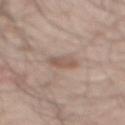<record>
  <biopsy_status>not biopsied; imaged during a skin examination</biopsy_status>
  <lighting>white-light</lighting>
  <lesion_size>
    <long_diameter_mm_approx>2.5</long_diameter_mm_approx>
  </lesion_size>
  <site>mid back</site>
  <patient>
    <sex>male</sex>
    <age_approx>50</age_approx>
  </patient>
  <image>
    <source>total-body photography crop</source>
    <field_of_view_mm>15</field_of_view_mm>
  </image>
</record>